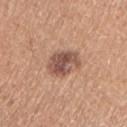Q: Was this lesion biopsied?
A: no biopsy performed (imaged during a skin exam)
Q: What are the patient's age and sex?
A: female, in their mid-60s
Q: What is the anatomic site?
A: the right upper arm
Q: What is the imaging modality?
A: ~15 mm tile from a whole-body skin photo